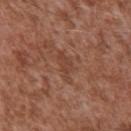Recorded during total-body skin imaging; not selected for excision or biopsy. Approximately 4 mm at its widest. This image is a 15 mm lesion crop taken from a total-body photograph. The lesion is on the chest. Imaged with white-light lighting. The patient is a male aged around 45.